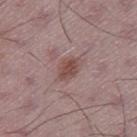Assessment:
Recorded during total-body skin imaging; not selected for excision or biopsy.
Acquisition and patient details:
Imaged with white-light lighting. This image is a 15 mm lesion crop taken from a total-body photograph. An algorithmic analysis of the crop reported a lesion area of about 6 mm² and a shape eccentricity near 0.75. And it measured a classifier nevus-likeness of about 70/100. A male patient roughly 50 years of age. From the leg.